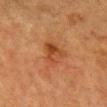Q: Was a biopsy performed?
A: imaged on a skin check; not biopsied
Q: How large is the lesion?
A: ≈3.5 mm
Q: How was the tile lit?
A: cross-polarized illumination
Q: Lesion location?
A: the head or neck
Q: How was this image acquired?
A: total-body-photography crop, ~15 mm field of view
Q: Automated lesion metrics?
A: an eccentricity of roughly 0.65 and a shape-asymmetry score of about 0.4 (0 = symmetric); a lesion color around L≈38 a*≈23 b*≈33 in CIELAB and roughly 7 lightness units darker than nearby skin; border irregularity of about 3.5 on a 0–10 scale, a color-variation rating of about 6.5/10, and radial color variation of about 2.5; an automated nevus-likeness rating near 5 out of 100 and lesion-presence confidence of about 100/100
Q: What are the patient's age and sex?
A: female, aged 53 to 57